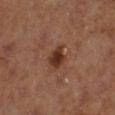illumination = cross-polarized; automated metrics = a classifier nevus-likeness of about 90/100 and a detector confidence of about 100 out of 100 that the crop contains a lesion; subject = female; lesion diameter = ≈2.5 mm; location = the right lower leg; image source = 15 mm crop, total-body photography.